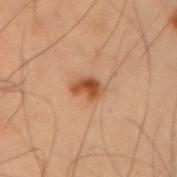  lesion_size:
    long_diameter_mm_approx: 3.0
  site: arm
  patient:
    sex: male
    age_approx: 55
  lighting: cross-polarized
  automated_metrics:
    cielab_L: 41
    cielab_a: 20
    cielab_b: 31
    vs_skin_darker_L: 11.0
    vs_skin_contrast_norm: 9.5
    lesion_detection_confidence_0_100: 100
  image:
    source: total-body photography crop
    field_of_view_mm: 15